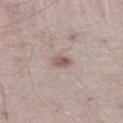Findings:
- follow-up: no biopsy performed (imaged during a skin exam)
- lesion diameter: about 2.5 mm
- body site: the right lower leg
- lighting: white-light
- image: total-body-photography crop, ~15 mm field of view
- subject: male, about 40 years old